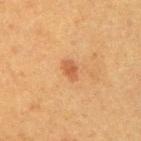The lesion was photographed on a routine skin check and not biopsied; there is no pathology result.
Longest diameter approximately 2.5 mm.
A female patient aged 38 to 42.
A roughly 15 mm field-of-view crop from a total-body skin photograph.
An algorithmic analysis of the crop reported a lesion area of about 3 mm². And it measured a mean CIELAB color near L≈47 a*≈22 b*≈34. It also reported a color-variation rating of about 1/10 and a peripheral color-asymmetry measure near 0.5. The analysis additionally found an automated nevus-likeness rating near 70 out of 100 and a detector confidence of about 100 out of 100 that the crop contains a lesion.
On the arm.